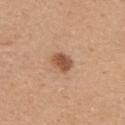Assessment: No biopsy was performed on this lesion — it was imaged during a full skin examination and was not determined to be concerning. Image and clinical context: A 15 mm crop from a total-body photograph taken for skin-cancer surveillance. A male subject aged approximately 40. This is a white-light tile. The lesion is located on the upper back. About 3 mm across.